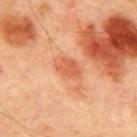Clinical impression:
The lesion was tiled from a total-body skin photograph and was not biopsied.
Image and clinical context:
Automated image analysis of the tile measured roughly 8 lightness units darker than nearby skin and a lesion-to-skin contrast of about 6 (normalized; higher = more distinct). The analysis additionally found an automated nevus-likeness rating near 0 out of 100 and a detector confidence of about 100 out of 100 that the crop contains a lesion. This is a cross-polarized tile. The recorded lesion diameter is about 3 mm. The lesion is on the chest. A male patient aged 63 to 67. A roughly 15 mm field-of-view crop from a total-body skin photograph.